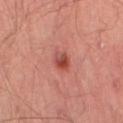Assessment:
No biopsy was performed on this lesion — it was imaged during a full skin examination and was not determined to be concerning.
Acquisition and patient details:
A male subject in their 70s. On the left thigh. An algorithmic analysis of the crop reported a footprint of about 4.5 mm², an outline eccentricity of about 0.6 (0 = round, 1 = elongated), and a symmetry-axis asymmetry near 0.2. It also reported an average lesion color of about L≈47 a*≈31 b*≈28 (CIELAB), a lesion–skin lightness drop of about 11, and a normalized lesion–skin contrast near 7.5. The analysis additionally found a border-irregularity index near 1.5/10, internal color variation of about 9.5 on a 0–10 scale, and radial color variation of about 3.5. The software also gave an automated nevus-likeness rating near 40 out of 100. About 2.5 mm across. The tile uses cross-polarized illumination. A roughly 15 mm field-of-view crop from a total-body skin photograph.